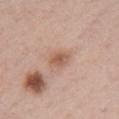Q: Was a biopsy performed?
A: imaged on a skin check; not biopsied
Q: How was this image acquired?
A: 15 mm crop, total-body photography
Q: What lighting was used for the tile?
A: white-light
Q: Lesion location?
A: the chest
Q: Who is the patient?
A: female, aged around 40
Q: What is the lesion's diameter?
A: ≈2.5 mm